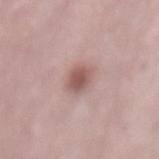Assessment: The lesion was tiled from a total-body skin photograph and was not biopsied. Acquisition and patient details: A male patient, roughly 50 years of age. A lesion tile, about 15 mm wide, cut from a 3D total-body photograph. The lesion is on the back. Approximately 3 mm at its widest. The total-body-photography lesion software estimated a border-irregularity index near 1.5/10 and radial color variation of about 0.5. The software also gave an automated nevus-likeness rating near 80 out of 100 and a lesion-detection confidence of about 100/100.